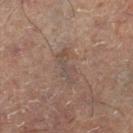The lesion was photographed on a routine skin check and not biopsied; there is no pathology result. A lesion tile, about 15 mm wide, cut from a 3D total-body photograph. A male patient, about 50 years old. The lesion is located on the right lower leg.